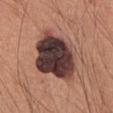This lesion was catalogued during total-body skin photography and was not selected for biopsy. A male patient, aged around 60. A 15 mm crop from a total-body photograph taken for skin-cancer surveillance. Measured at roughly 6.5 mm in maximum diameter. Located on the chest. Imaged with white-light lighting.